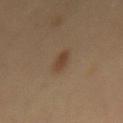- biopsy status — no biopsy performed (imaged during a skin exam)
- image-analysis metrics — an area of roughly 4 mm², an outline eccentricity of about 0.9 (0 = round, 1 = elongated), and a shape-asymmetry score of about 0.4 (0 = symmetric); an average lesion color of about L≈41 a*≈16 b*≈28 (CIELAB), about 8 CIELAB-L* units darker than the surrounding skin, and a normalized lesion–skin contrast near 7
- image — 15 mm crop, total-body photography
- patient — male, about 40 years old
- lesion diameter — about 3.5 mm
- location — the mid back
- illumination — cross-polarized illumination